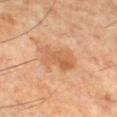workup — catalogued during a skin exam; not biopsied
location — the leg
patient — male, in their 60s
lighting — cross-polarized
image — total-body-photography crop, ~15 mm field of view